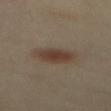Impression: Captured during whole-body skin photography for melanoma surveillance; the lesion was not biopsied. Context: A female patient, in their 40s. Imaged with cross-polarized lighting. Longest diameter approximately 4 mm. Located on the mid back. The total-body-photography lesion software estimated a lesion area of about 8 mm², an outline eccentricity of about 0.7 (0 = round, 1 = elongated), and a shape-asymmetry score of about 0.15 (0 = symmetric). The software also gave about 9 CIELAB-L* units darker than the surrounding skin and a normalized lesion–skin contrast near 8.5. The analysis additionally found a nevus-likeness score of about 100/100 and lesion-presence confidence of about 100/100. A region of skin cropped from a whole-body photographic capture, roughly 15 mm wide.